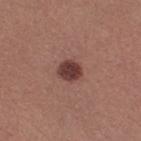biopsy status=no biopsy performed (imaged during a skin exam); image=15 mm crop, total-body photography; patient=female, aged around 25; tile lighting=white-light illumination; body site=the leg; TBP lesion metrics=a footprint of about 5.5 mm² and two-axis asymmetry of about 0.15; diameter=about 3 mm.